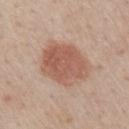Background: A male patient aged approximately 60. Automated tile analysis of the lesion measured border irregularity of about 1.5 on a 0–10 scale and a color-variation rating of about 3.5/10. This image is a 15 mm lesion crop taken from a total-body photograph. About 6 mm across. Located on the mid back.